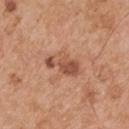The lesion was tiled from a total-body skin photograph and was not biopsied.
This is a white-light tile.
On the arm.
Automated tile analysis of the lesion measured an average lesion color of about L≈52 a*≈24 b*≈32 (CIELAB) and a lesion-to-skin contrast of about 7.5 (normalized; higher = more distinct).
The recorded lesion diameter is about 4.5 mm.
The subject is a male aged around 55.
A region of skin cropped from a whole-body photographic capture, roughly 15 mm wide.